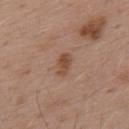Clinical summary:
Cropped from a total-body skin-imaging series; the visible field is about 15 mm. The lesion is on the upper back. The subject is a male approximately 55 years of age.